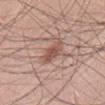workup — catalogued during a skin exam; not biopsied | subject — male, aged approximately 60 | site — the back | size — about 4 mm | tile lighting — white-light illumination | automated lesion analysis — a mean CIELAB color near L≈53 a*≈21 b*≈25, a lesion–skin lightness drop of about 10, and a normalized border contrast of about 7; a border-irregularity index near 2.5/10, a color-variation rating of about 3/10, and radial color variation of about 1 | image — ~15 mm crop, total-body skin-cancer survey.